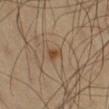Q: Was this lesion biopsied?
A: total-body-photography surveillance lesion; no biopsy
Q: Lesion location?
A: the right thigh
Q: Patient demographics?
A: male, roughly 60 years of age
Q: What did automated image analysis measure?
A: an automated nevus-likeness rating near 90 out of 100
Q: How was the tile lit?
A: cross-polarized
Q: How was this image acquired?
A: total-body-photography crop, ~15 mm field of view